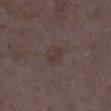No biopsy was performed on this lesion — it was imaged during a full skin examination and was not determined to be concerning. From the left lower leg. A 15 mm crop from a total-body photograph taken for skin-cancer surveillance. This is a white-light tile. Automated image analysis of the tile measured an area of roughly 5 mm². And it measured a mean CIELAB color near L≈36 a*≈13 b*≈19, about 4 CIELAB-L* units darker than the surrounding skin, and a normalized lesion–skin contrast near 5.5. The analysis additionally found an automated nevus-likeness rating near 0 out of 100. A female subject approximately 35 years of age. Approximately 3 mm at its widest.